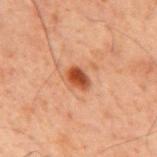Notes:
• lesion size — ~2.5 mm (longest diameter)
• location — the mid back
• image source — 15 mm crop, total-body photography
• patient — male, about 60 years old